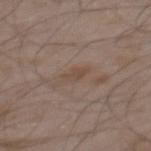This lesion was catalogued during total-body skin photography and was not selected for biopsy. The lesion's longest dimension is about 2.5 mm. A roughly 15 mm field-of-view crop from a total-body skin photograph. Captured under white-light illumination. The lesion is on the upper back. A male subject about 55 years old. Automated image analysis of the tile measured a lesion area of about 3 mm², an eccentricity of roughly 0.85, and two-axis asymmetry of about 0.25. It also reported a mean CIELAB color near L≈46 a*≈14 b*≈24 and about 6 CIELAB-L* units darker than the surrounding skin.